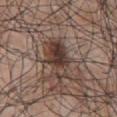The lesion was photographed on a routine skin check and not biopsied; there is no pathology result. From the chest. This is a white-light tile. The subject is a male in their 70s. About 5 mm across. A lesion tile, about 15 mm wide, cut from a 3D total-body photograph.